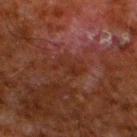follow-up — no biopsy performed (imaged during a skin exam)
imaging modality — total-body-photography crop, ~15 mm field of view
automated metrics — a lesion area of about 3.5 mm² and an eccentricity of roughly 0.9; a mean CIELAB color near L≈22 a*≈20 b*≈23; a border-irregularity index near 7.5/10, a within-lesion color-variation index near 1/10, and radial color variation of about 0.5
illumination — cross-polarized illumination
anatomic site — the left lower leg
subject — male, aged around 80